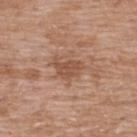Findings:
– workup · no biopsy performed (imaged during a skin exam)
– lesion size · ~3.5 mm (longest diameter)
– location · the upper back
– subject · male, approximately 50 years of age
– imaging modality · ~15 mm crop, total-body skin-cancer survey
– automated lesion analysis · a normalized border contrast of about 6.5; a border-irregularity rating of about 4.5/10, internal color variation of about 1.5 on a 0–10 scale, and peripheral color asymmetry of about 0.5
– illumination · white-light